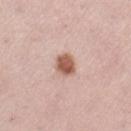Recorded during total-body skin imaging; not selected for excision or biopsy.
The lesion is on the left thigh.
A female subject aged 43 to 47.
A close-up tile cropped from a whole-body skin photograph, about 15 mm across.
Approximately 3 mm at its widest.
This is a white-light tile.